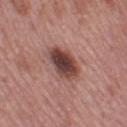Q: Is there a histopathology result?
A: imaged on a skin check; not biopsied
Q: How was this image acquired?
A: 15 mm crop, total-body photography
Q: Lesion size?
A: ~4.5 mm (longest diameter)
Q: Who is the patient?
A: female, about 50 years old
Q: What is the anatomic site?
A: the leg